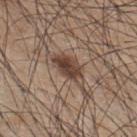imaging modality = 15 mm crop, total-body photography
tile lighting = white-light
image-analysis metrics = a border-irregularity index near 3.5/10, a color-variation rating of about 5/10, and peripheral color asymmetry of about 2; an automated nevus-likeness rating near 80 out of 100 and a lesion-detection confidence of about 100/100
patient = male, in their mid- to late 40s
site = the upper back
lesion size = ~5 mm (longest diameter)